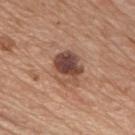Impression: Imaged during a routine full-body skin examination; the lesion was not biopsied and no histopathology is available. Image and clinical context: A 15 mm crop from a total-body photograph taken for skin-cancer surveillance. The subject is a male aged around 75. The lesion's longest dimension is about 4 mm. The lesion is located on the chest. Imaged with white-light lighting.